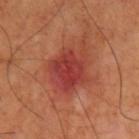illumination = cross-polarized illumination
anatomic site = the left upper arm
patient = male, roughly 70 years of age
lesion size = ~5.5 mm (longest diameter)
imaging modality = ~15 mm crop, total-body skin-cancer survey
pathology = a nodular basal cell carcinoma